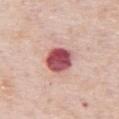biopsy_status: not biopsied; imaged during a skin examination